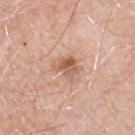No biopsy was performed on this lesion — it was imaged during a full skin examination and was not determined to be concerning.
The tile uses white-light illumination.
The subject is a male approximately 70 years of age.
A close-up tile cropped from a whole-body skin photograph, about 15 mm across.
Located on the chest.
The lesion's longest dimension is about 3 mm.